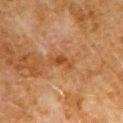The lesion was tiled from a total-body skin photograph and was not biopsied. Approximately 3 mm at its widest. Located on the left upper arm. A 15 mm close-up tile from a total-body photography series done for melanoma screening. Captured under cross-polarized illumination. A male subject, roughly 80 years of age.